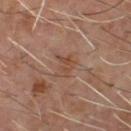Recorded during total-body skin imaging; not selected for excision or biopsy.
From the front of the torso.
Captured under cross-polarized illumination.
The lesion's longest dimension is about 3 mm.
Cropped from a total-body skin-imaging series; the visible field is about 15 mm.
A male subject approximately 60 years of age.